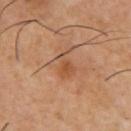biopsy status=imaged on a skin check; not biopsied | patient=male, aged 53–57 | automated metrics=a lesion area of about 5.5 mm², an eccentricity of roughly 0.8, and two-axis asymmetry of about 0.4; a within-lesion color-variation index near 3/10 and peripheral color asymmetry of about 1; lesion-presence confidence of about 100/100 | acquisition=15 mm crop, total-body photography | anatomic site=the chest | size=~3.5 mm (longest diameter) | illumination=cross-polarized.